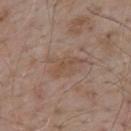follow-up=no biopsy performed (imaged during a skin exam) | imaging modality=~15 mm tile from a whole-body skin photo | lesion size=≈4.5 mm | body site=the upper back | lighting=white-light illumination | patient=male, aged approximately 55.